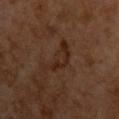Assessment: The lesion was photographed on a routine skin check and not biopsied; there is no pathology result. Image and clinical context: Measured at roughly 5 mm in maximum diameter. This is a cross-polarized tile. From the front of the torso. A male subject, approximately 60 years of age. A 15 mm close-up extracted from a 3D total-body photography capture. Automated tile analysis of the lesion measured an average lesion color of about L≈22 a*≈17 b*≈23 (CIELAB) and a normalized border contrast of about 7. The software also gave a within-lesion color-variation index near 0.5/10.